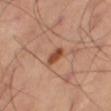tile lighting — cross-polarized | acquisition — 15 mm crop, total-body photography | location — the right thigh.